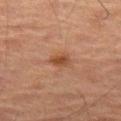  biopsy_status: not biopsied; imaged during a skin examination